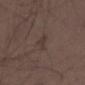<case>
<biopsy_status>not biopsied; imaged during a skin examination</biopsy_status>
<site>right thigh</site>
<lighting>white-light</lighting>
<image>
  <source>total-body photography crop</source>
  <field_of_view_mm>15</field_of_view_mm>
</image>
<lesion_size>
  <long_diameter_mm_approx>2.5</long_diameter_mm_approx>
</lesion_size>
<patient>
  <sex>male</sex>
  <age_approx>50</age_approx>
</patient>
</case>